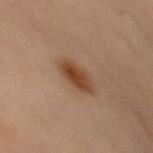Assessment:
Part of a total-body skin-imaging series; this lesion was reviewed on a skin check and was not flagged for biopsy.
Image and clinical context:
The patient is a female aged approximately 60. The lesion is on the upper back. The tile uses cross-polarized illumination. Longest diameter approximately 4 mm. A region of skin cropped from a whole-body photographic capture, roughly 15 mm wide.